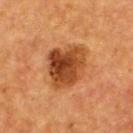This lesion was catalogued during total-body skin photography and was not selected for biopsy. The recorded lesion diameter is about 5 mm. Cropped from a total-body skin-imaging series; the visible field is about 15 mm. A female subject, in their mid-50s. On the upper back.